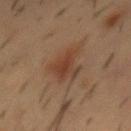Assessment:
This lesion was catalogued during total-body skin photography and was not selected for biopsy.
Image and clinical context:
A region of skin cropped from a whole-body photographic capture, roughly 15 mm wide. From the mid back. The total-body-photography lesion software estimated a lesion area of about 9 mm² and two-axis asymmetry of about 0.45. The software also gave a border-irregularity rating of about 5.5/10. The software also gave an automated nevus-likeness rating near 90 out of 100. A male subject approximately 55 years of age. Longest diameter approximately 4.5 mm.